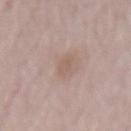Case summary:
– workup: total-body-photography surveillance lesion; no biopsy
– patient: male, in their mid-60s
– site: the lower back
– acquisition: total-body-photography crop, ~15 mm field of view